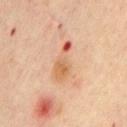No biopsy was performed on this lesion — it was imaged during a full skin examination and was not determined to be concerning.
A close-up tile cropped from a whole-body skin photograph, about 15 mm across.
The recorded lesion diameter is about 5 mm.
This is a cross-polarized tile.
A female subject, aged approximately 45.
Located on the chest.